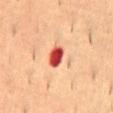biopsy_status: not biopsied; imaged during a skin examination
image:
  source: total-body photography crop
  field_of_view_mm: 15
site: mid back
lesion_size:
  long_diameter_mm_approx: 3.0
automated_metrics:
  area_mm2_approx: 4.5
  eccentricity: 0.75
  shape_asymmetry: 0.15
  cielab_L: 45
  cielab_a: 39
  cielab_b: 32
  vs_skin_darker_L: 21.0
  vs_skin_contrast_norm: 14.0
  nevus_likeness_0_100: 0
patient:
  sex: male
  age_approx: 55
lighting: cross-polarized